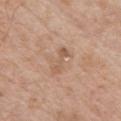Notes:
- follow-up · imaged on a skin check; not biopsied
- anatomic site · the chest
- diameter · ~3.5 mm (longest diameter)
- acquisition · ~15 mm tile from a whole-body skin photo
- automated lesion analysis · a lesion area of about 3.5 mm², an eccentricity of roughly 0.95, and a symmetry-axis asymmetry near 0.3; an average lesion color of about L≈57 a*≈19 b*≈31 (CIELAB), a lesion–skin lightness drop of about 8, and a normalized lesion–skin contrast near 5.5; a border-irregularity index near 4.5/10, a within-lesion color-variation index near 0/10, and peripheral color asymmetry of about 0; an automated nevus-likeness rating near 0 out of 100
- subject · male, approximately 65 years of age